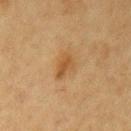Assessment: Part of a total-body skin-imaging series; this lesion was reviewed on a skin check and was not flagged for biopsy. Image and clinical context: The lesion is on the left upper arm. Automated tile analysis of the lesion measured an average lesion color of about L≈44 a*≈18 b*≈35 (CIELAB), a lesion–skin lightness drop of about 8, and a lesion-to-skin contrast of about 7 (normalized; higher = more distinct). The software also gave border irregularity of about 4 on a 0–10 scale and radial color variation of about 1. It also reported an automated nevus-likeness rating near 30 out of 100. This image is a 15 mm lesion crop taken from a total-body photograph. The patient is a female aged 48 to 52.